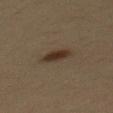| feature | finding |
|---|---|
| biopsy status | catalogued during a skin exam; not biopsied |
| lesion size | about 3 mm |
| site | the mid back |
| patient | male, aged around 35 |
| acquisition | ~15 mm tile from a whole-body skin photo |